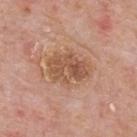• follow-up — total-body-photography surveillance lesion; no biopsy
• site — the back
• image source — ~15 mm crop, total-body skin-cancer survey
• lesion diameter — about 5 mm
• lighting — white-light
• subject — male, aged around 75